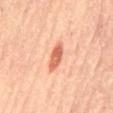Q: Was this lesion biopsied?
A: no biopsy performed (imaged during a skin exam)
Q: Lesion location?
A: the abdomen
Q: How was this image acquired?
A: total-body-photography crop, ~15 mm field of view
Q: What are the patient's age and sex?
A: male, aged 63–67